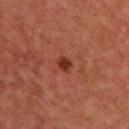biopsy status = total-body-photography surveillance lesion; no biopsy | image = 15 mm crop, total-body photography | location = the back | patient = male, aged approximately 65.